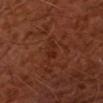{"image": {"source": "total-body photography crop", "field_of_view_mm": 15}, "patient": {"sex": "male", "age_approx": 65}, "lesion_size": {"long_diameter_mm_approx": 3.0}, "lighting": "cross-polarized"}